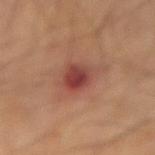A male subject, approximately 70 years of age. A close-up tile cropped from a whole-body skin photograph, about 15 mm across. From the left forearm. Automated image analysis of the tile measured a footprint of about 12 mm², a shape eccentricity near 0.7, and two-axis asymmetry of about 0.3. It also reported an average lesion color of about L≈45 a*≈25 b*≈26 (CIELAB), roughly 10 lightness units darker than nearby skin, and a normalized border contrast of about 8.